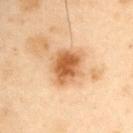biopsy status — catalogued during a skin exam; not biopsied
site — the left upper arm
automated metrics — a lesion area of about 13 mm² and an outline eccentricity of about 0.5 (0 = round, 1 = elongated); border irregularity of about 2.5 on a 0–10 scale and peripheral color asymmetry of about 1
patient — male, aged 53 to 57
lesion diameter — ≈4 mm
acquisition — total-body-photography crop, ~15 mm field of view